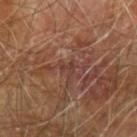patient: male, aged 68–72
body site: the left forearm
image-analysis metrics: a lesion area of about 2 mm², an eccentricity of roughly 0.65, and two-axis asymmetry of about 0.55; a classifier nevus-likeness of about 0/100 and a lesion-detection confidence of about 10/100
illumination: cross-polarized
imaging modality: 15 mm crop, total-body photography
diameter: about 2 mm